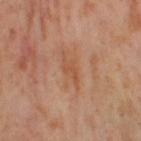Captured during whole-body skin photography for melanoma surveillance; the lesion was not biopsied.
A female subject, about 55 years old.
A 15 mm crop from a total-body photograph taken for skin-cancer surveillance.
An algorithmic analysis of the crop reported a lesion color around L≈53 a*≈24 b*≈34 in CIELAB and a lesion-to-skin contrast of about 5 (normalized; higher = more distinct).
Located on the right thigh.
The lesion's longest dimension is about 3 mm.